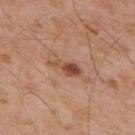Q: Is there a histopathology result?
A: catalogued during a skin exam; not biopsied
Q: How was the tile lit?
A: white-light illumination
Q: What are the patient's age and sex?
A: male, aged 53 to 57
Q: What is the imaging modality?
A: 15 mm crop, total-body photography
Q: How large is the lesion?
A: ≈4 mm
Q: What did automated image analysis measure?
A: a footprint of about 5 mm², an eccentricity of roughly 0.9, and a shape-asymmetry score of about 0.4 (0 = symmetric); an average lesion color of about L≈50 a*≈22 b*≈31 (CIELAB) and about 11 CIELAB-L* units darker than the surrounding skin; a lesion-detection confidence of about 100/100
Q: Where on the body is the lesion?
A: the upper back